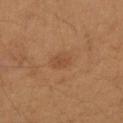notes: no biopsy performed (imaged during a skin exam)
subject: female, approximately 30 years of age
automated metrics: an average lesion color of about L≈45 a*≈20 b*≈32 (CIELAB) and a lesion-to-skin contrast of about 4.5 (normalized; higher = more distinct); a border-irregularity index near 2/10 and internal color variation of about 2 on a 0–10 scale; a classifier nevus-likeness of about 20/100 and a lesion-detection confidence of about 100/100
imaging modality: 15 mm crop, total-body photography
body site: the arm